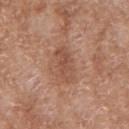{"biopsy_status": "not biopsied; imaged during a skin examination", "image": {"source": "total-body photography crop", "field_of_view_mm": 15}, "site": "arm", "patient": {"sex": "female", "age_approx": 75}}